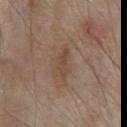Findings:
– workup: catalogued during a skin exam; not biopsied